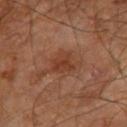<record>
  <biopsy_status>not biopsied; imaged during a skin examination</biopsy_status>
  <automated_metrics>
    <cielab_L>37</cielab_L>
    <cielab_a>23</cielab_a>
    <cielab_b>30</cielab_b>
    <vs_skin_darker_L>7.0</vs_skin_darker_L>
    <vs_skin_contrast_norm>7.0</vs_skin_contrast_norm>
    <border_irregularity_0_10>3.5</border_irregularity_0_10>
    <color_variation_0_10>3.0</color_variation_0_10>
    <peripheral_color_asymmetry>1.0</peripheral_color_asymmetry>
  </automated_metrics>
  <patient>
    <sex>male</sex>
    <age_approx>60</age_approx>
  </patient>
  <image>
    <source>total-body photography crop</source>
    <field_of_view_mm>15</field_of_view_mm>
  </image>
  <lighting>cross-polarized</lighting>
  <site>leg</site>
</record>